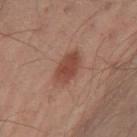Assessment:
Part of a total-body skin-imaging series; this lesion was reviewed on a skin check and was not flagged for biopsy.
Acquisition and patient details:
A 15 mm close-up extracted from a 3D total-body photography capture. A male patient in their 50s. On the left leg.